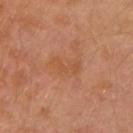Clinical impression:
This lesion was catalogued during total-body skin photography and was not selected for biopsy.
Image and clinical context:
A male patient aged approximately 30. Longest diameter approximately 3.5 mm. A close-up tile cropped from a whole-body skin photograph, about 15 mm across. An algorithmic analysis of the crop reported a mean CIELAB color near L≈50 a*≈24 b*≈35 and a normalized border contrast of about 5. And it measured a border-irregularity rating of about 6/10 and a color-variation rating of about 1.5/10. The lesion is on the left arm.